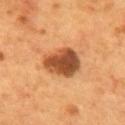Imaged during a routine full-body skin examination; the lesion was not biopsied and no histopathology is available. Cropped from a total-body skin-imaging series; the visible field is about 15 mm. This is a cross-polarized tile. A male patient, about 55 years old. Located on the back. Automated tile analysis of the lesion measured a lesion area of about 13 mm², an eccentricity of roughly 0.65, and two-axis asymmetry of about 0.2. The software also gave a lesion color around L≈46 a*≈25 b*≈36 in CIELAB, a lesion–skin lightness drop of about 16, and a normalized border contrast of about 11.5. The analysis additionally found border irregularity of about 2 on a 0–10 scale.